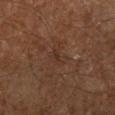Cropped from a whole-body photographic skin survey; the tile spans about 15 mm.
The lesion is on the right lower leg.
The patient is a male aged 58–62.